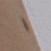Recorded during total-body skin imaging; not selected for excision or biopsy. A female patient, aged 38 to 42. Cropped from a whole-body photographic skin survey; the tile spans about 15 mm. From the right upper arm.